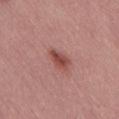Clinical impression: Captured during whole-body skin photography for melanoma surveillance; the lesion was not biopsied. Context: The tile uses white-light illumination. A region of skin cropped from a whole-body photographic capture, roughly 15 mm wide. The subject is a male aged around 55. On the lower back.